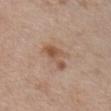Part of a total-body skin-imaging series; this lesion was reviewed on a skin check and was not flagged for biopsy. The total-body-photography lesion software estimated an outline eccentricity of about 0.85 (0 = round, 1 = elongated) and two-axis asymmetry of about 0.45. The software also gave a nevus-likeness score of about 15/100 and lesion-presence confidence of about 100/100. Imaged with white-light lighting. A female subject roughly 40 years of age. Cropped from a whole-body photographic skin survey; the tile spans about 15 mm. Located on the chest.